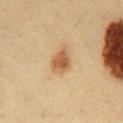lighting: cross-polarized
image:
  source: total-body photography crop
  field_of_view_mm: 15
site: chest
patient:
  sex: male
  age_approx: 35
automated_metrics:
  border_irregularity_0_10: 2.5
  peripheral_color_asymmetry: 1.0